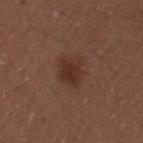This lesion was catalogued during total-body skin photography and was not selected for biopsy.
The tile uses white-light illumination.
The lesion is located on the right upper arm.
A male subject, roughly 30 years of age.
Cropped from a whole-body photographic skin survey; the tile spans about 15 mm.
The total-body-photography lesion software estimated a lesion area of about 7 mm² and a symmetry-axis asymmetry near 0.2. The analysis additionally found roughly 8 lightness units darker than nearby skin.
The lesion's longest dimension is about 3.5 mm.